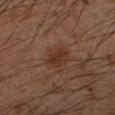{
  "biopsy_status": "not biopsied; imaged during a skin examination",
  "patient": {
    "sex": "male",
    "age_approx": 55
  },
  "site": "left forearm",
  "image": {
    "source": "total-body photography crop",
    "field_of_view_mm": 15
  }
}